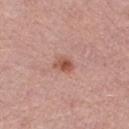{"biopsy_status": "not biopsied; imaged during a skin examination", "patient": {"sex": "female", "age_approx": 70}, "image": {"source": "total-body photography crop", "field_of_view_mm": 15}, "site": "left thigh"}